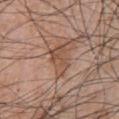| field | value |
|---|---|
| biopsy status | no biopsy performed (imaged during a skin exam) |
| location | the front of the torso |
| automated metrics | an outline eccentricity of about 0.8 (0 = round, 1 = elongated) and a symmetry-axis asymmetry near 0.35; a lesion color around L≈50 a*≈19 b*≈29 in CIELAB and a normalized border contrast of about 6.5; border irregularity of about 4.5 on a 0–10 scale, internal color variation of about 3.5 on a 0–10 scale, and radial color variation of about 1; a classifier nevus-likeness of about 0/100 and a detector confidence of about 95 out of 100 that the crop contains a lesion |
| imaging modality | 15 mm crop, total-body photography |
| lesion size | ~4 mm (longest diameter) |
| subject | male, aged around 70 |